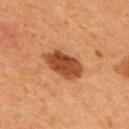Findings:
• workup · imaged on a skin check; not biopsied
• site · the mid back
• subject · male, aged 53–57
• image source · ~15 mm tile from a whole-body skin photo
• lighting · cross-polarized illumination
• size · about 5 mm